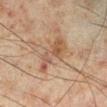notes=no biopsy performed (imaged during a skin exam)
image=~15 mm crop, total-body skin-cancer survey
patient=male, in their mid- to late 40s
body site=the right lower leg